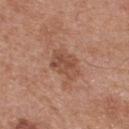{
  "biopsy_status": "not biopsied; imaged during a skin examination",
  "patient": {
    "sex": "male",
    "age_approx": 30
  },
  "lighting": "white-light",
  "lesion_size": {
    "long_diameter_mm_approx": 3.5
  },
  "image": {
    "source": "total-body photography crop",
    "field_of_view_mm": 15
  },
  "site": "upper back"
}